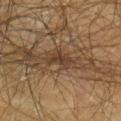Assessment: Imaged during a routine full-body skin examination; the lesion was not biopsied and no histopathology is available. Background: Automated image analysis of the tile measured a border-irregularity index near 4/10 and peripheral color asymmetry of about 0.5. The software also gave an automated nevus-likeness rating near 0 out of 100 and lesion-presence confidence of about 55/100. The subject is a male aged around 50. Imaged with cross-polarized lighting. The lesion is on the right lower leg. A region of skin cropped from a whole-body photographic capture, roughly 15 mm wide. Measured at roughly 3 mm in maximum diameter.